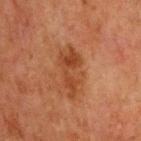Q: Is there a histopathology result?
A: total-body-photography surveillance lesion; no biopsy
Q: What are the patient's age and sex?
A: male, in their mid- to late 60s
Q: What is the lesion's diameter?
A: ~5.5 mm (longest diameter)
Q: What kind of image is this?
A: 15 mm crop, total-body photography
Q: What lighting was used for the tile?
A: cross-polarized illumination
Q: Lesion location?
A: the chest